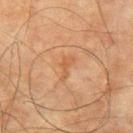{
  "image": {
    "source": "total-body photography crop",
    "field_of_view_mm": 15
  },
  "patient": {
    "sex": "male",
    "age_approx": 75
  },
  "site": "right upper arm"
}